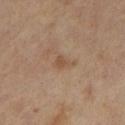The lesion is on the leg. This image is a 15 mm lesion crop taken from a total-body photograph. A female patient, aged around 65.